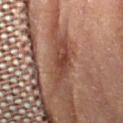This lesion was catalogued during total-body skin photography and was not selected for biopsy.
The subject is a male about 85 years old or older.
The lesion is on the right forearm.
A region of skin cropped from a whole-body photographic capture, roughly 15 mm wide.
Measured at roughly 6 mm in maximum diameter.
Automated tile analysis of the lesion measured a footprint of about 10 mm² and a shape eccentricity near 0.9. The software also gave a classifier nevus-likeness of about 0/100 and a lesion-detection confidence of about 85/100.
Imaged with cross-polarized lighting.